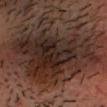  biopsy_status: not biopsied; imaged during a skin examination
  patient:
    sex: male
    age_approx: 35
  site: head or neck
  lesion_size:
    long_diameter_mm_approx: 11.0
  automated_metrics:
    area_mm2_approx: 75.0
    eccentricity: 0.35
    shape_asymmetry: 0.35
    lesion_detection_confidence_0_100: 75
  image:
    source: total-body photography crop
    field_of_view_mm: 15